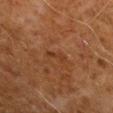The lesion was tiled from a total-body skin photograph and was not biopsied. A male patient, roughly 70 years of age. On the arm. A 15 mm close-up extracted from a 3D total-body photography capture. Automated tile analysis of the lesion measured a footprint of about 2.5 mm² and an outline eccentricity of about 0.95 (0 = round, 1 = elongated). The analysis additionally found a mean CIELAB color near L≈30 a*≈20 b*≈29, about 5 CIELAB-L* units darker than the surrounding skin, and a lesion-to-skin contrast of about 5.5 (normalized; higher = more distinct). And it measured a nevus-likeness score of about 0/100 and lesion-presence confidence of about 100/100. The tile uses cross-polarized illumination.